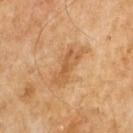Q: Is there a histopathology result?
A: no biopsy performed (imaged during a skin exam)
Q: What is the imaging modality?
A: ~15 mm tile from a whole-body skin photo
Q: Who is the patient?
A: male, in their mid- to late 60s